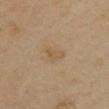biopsy status — no biopsy performed (imaged during a skin exam)
tile lighting — cross-polarized illumination
size — about 2.5 mm
subject — female, aged 33–37
imaging modality — 15 mm crop, total-body photography
automated lesion analysis — an area of roughly 3.5 mm², a shape eccentricity near 0.7, and two-axis asymmetry of about 0.5; a lesion–skin lightness drop of about 5 and a lesion-to-skin contrast of about 5 (normalized; higher = more distinct); an automated nevus-likeness rating near 0 out of 100 and a detector confidence of about 100 out of 100 that the crop contains a lesion
body site — the chest